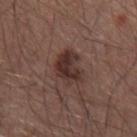| feature | finding |
|---|---|
| notes | catalogued during a skin exam; not biopsied |
| site | the right upper arm |
| image | ~15 mm tile from a whole-body skin photo |
| subject | male, aged 53–57 |
| lighting | white-light |
| lesion diameter | about 4 mm |